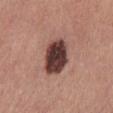notes: catalogued during a skin exam; not biopsied | illumination: white-light illumination | image: total-body-photography crop, ~15 mm field of view | location: the mid back | automated metrics: an area of roughly 13 mm², a shape eccentricity near 0.65, and a shape-asymmetry score of about 0.15 (0 = symmetric); an average lesion color of about L≈39 a*≈21 b*≈21 (CIELAB) and a normalized border contrast of about 15.5; a border-irregularity index near 1.5/10, internal color variation of about 5 on a 0–10 scale, and radial color variation of about 1.5 | size: about 4.5 mm | patient: male, aged 38 to 42.